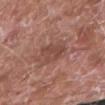Imaged during a routine full-body skin examination; the lesion was not biopsied and no histopathology is available. A male patient in their 80s. The total-body-photography lesion software estimated a lesion–skin lightness drop of about 7 and a lesion-to-skin contrast of about 5.5 (normalized; higher = more distinct). It also reported a border-irregularity index near 3/10, a color-variation rating of about 2.5/10, and radial color variation of about 1. The software also gave a nevus-likeness score of about 0/100 and a lesion-detection confidence of about 100/100. The lesion's longest dimension is about 3.5 mm. A close-up tile cropped from a whole-body skin photograph, about 15 mm across. From the right forearm.